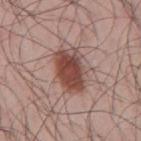biopsy_status: not biopsied; imaged during a skin examination
lesion_size:
  long_diameter_mm_approx: 5.5
automated_metrics:
  area_mm2_approx: 14.0
  eccentricity: 0.8
patient:
  sex: male
  age_approx: 45
lighting: white-light
image:
  source: total-body photography crop
  field_of_view_mm: 15
site: mid back